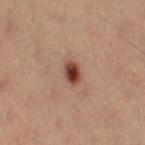follow-up: imaged on a skin check; not biopsied | site: the right thigh | imaging modality: total-body-photography crop, ~15 mm field of view | illumination: cross-polarized illumination | automated metrics: a mean CIELAB color near L≈43 a*≈23 b*≈28, roughly 16 lightness units darker than nearby skin, and a normalized lesion–skin contrast near 12; border irregularity of about 1.5 on a 0–10 scale and peripheral color asymmetry of about 2; an automated nevus-likeness rating near 100 out of 100 and a lesion-detection confidence of about 100/100 | subject: female, aged around 35 | lesion size: ~3 mm (longest diameter).